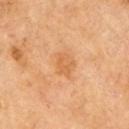Case summary:
• workup — imaged on a skin check; not biopsied
• lesion size — about 3 mm
• site — the right upper arm
• tile lighting — cross-polarized illumination
• acquisition — total-body-photography crop, ~15 mm field of view
• patient — male, roughly 70 years of age